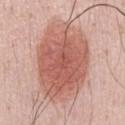subject=male, aged 48 to 52 | location=the chest | lighting=white-light illumination | image=~15 mm crop, total-body skin-cancer survey.